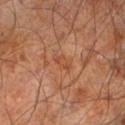This lesion was catalogued during total-body skin photography and was not selected for biopsy.